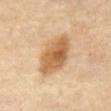| key | value |
|---|---|
| workup | catalogued during a skin exam; not biopsied |
| tile lighting | cross-polarized |
| subject | male, aged approximately 70 |
| location | the front of the torso |
| image source | total-body-photography crop, ~15 mm field of view |